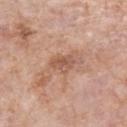Q: Is there a histopathology result?
A: total-body-photography surveillance lesion; no biopsy
Q: Lesion location?
A: the left forearm
Q: What is the imaging modality?
A: total-body-photography crop, ~15 mm field of view
Q: How large is the lesion?
A: ~2.5 mm (longest diameter)
Q: Illumination type?
A: white-light
Q: What did automated image analysis measure?
A: an outline eccentricity of about 0.6 (0 = round, 1 = elongated); a classifier nevus-likeness of about 0/100 and lesion-presence confidence of about 100/100
Q: What are the patient's age and sex?
A: female, in their 70s